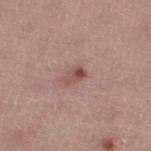{"biopsy_status": "not biopsied; imaged during a skin examination", "site": "left lower leg", "lesion_size": {"long_diameter_mm_approx": 2.5}, "image": {"source": "total-body photography crop", "field_of_view_mm": 15}, "lighting": "white-light", "patient": {"sex": "female", "age_approx": 45}}